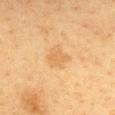Acquisition and patient details: Located on the chest. A lesion tile, about 15 mm wide, cut from a 3D total-body photograph. Captured under cross-polarized illumination. A female patient about 40 years old.